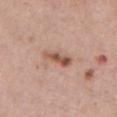The lesion was tiled from a total-body skin photograph and was not biopsied. The lesion-visualizer software estimated an area of roughly 5 mm², an outline eccentricity of about 0.9 (0 = round, 1 = elongated), and a symmetry-axis asymmetry near 0.3. The analysis additionally found a border-irregularity rating of about 3.5/10, a within-lesion color-variation index near 4/10, and radial color variation of about 1.5. The analysis additionally found a classifier nevus-likeness of about 85/100 and lesion-presence confidence of about 100/100. A roughly 15 mm field-of-view crop from a total-body skin photograph. A female patient aged approximately 55. Located on the chest.